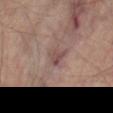Case summary:
• notes — imaged on a skin check; not biopsied
• tile lighting — cross-polarized illumination
• automated metrics — a border-irregularity rating of about 3.5/10 and radial color variation of about 1
• lesion size — ~2.5 mm (longest diameter)
• location — the right thigh
• image source — total-body-photography crop, ~15 mm field of view
• patient — male, aged around 70